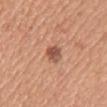{"biopsy_status": "not biopsied; imaged during a skin examination", "patient": {"sex": "female", "age_approx": 45}, "image": {"source": "total-body photography crop", "field_of_view_mm": 15}, "automated_metrics": {"area_mm2_approx": 4.5, "eccentricity": 0.65, "shape_asymmetry": 0.2, "cielab_L": 53, "cielab_a": 24, "cielab_b": 31, "vs_skin_contrast_norm": 8.5, "border_irregularity_0_10": 2.0, "color_variation_0_10": 3.5, "nevus_likeness_0_100": 70, "lesion_detection_confidence_0_100": 100}, "lighting": "white-light", "site": "right upper arm"}